The total-body-photography lesion software estimated a normalized border contrast of about 8. It also reported border irregularity of about 3.5 on a 0–10 scale and radial color variation of about 1.5. The software also gave a nevus-likeness score of about 30/100 and a detector confidence of about 100 out of 100 that the crop contains a lesion; this is a white-light tile; a female patient aged 43 to 47; the lesion is on the upper back; a 15 mm close-up extracted from a 3D total-body photography capture; the lesion's longest dimension is about 6 mm: 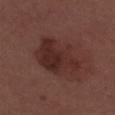Case summary:
- biopsy diagnosis: an intradermal melanocytic nevus (benign)